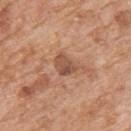Q: Was this lesion biopsied?
A: imaged on a skin check; not biopsied
Q: What is the imaging modality?
A: ~15 mm tile from a whole-body skin photo
Q: Lesion size?
A: ≈3 mm
Q: What lighting was used for the tile?
A: white-light
Q: Patient demographics?
A: male, aged approximately 60
Q: Where on the body is the lesion?
A: the back